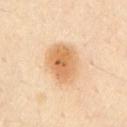Case summary:
• imaging modality: 15 mm crop, total-body photography
• image-analysis metrics: a nevus-likeness score of about 95/100 and lesion-presence confidence of about 100/100
• anatomic site: the chest
• patient: male, roughly 70 years of age
• diameter: ≈5 mm